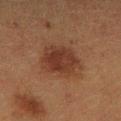Part of a total-body skin-imaging series; this lesion was reviewed on a skin check and was not flagged for biopsy.